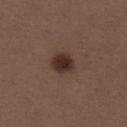Notes:
* follow-up: imaged on a skin check; not biopsied
* subject: female, aged approximately 40
* imaging modality: 15 mm crop, total-body photography
* lesion diameter: ~2.5 mm (longest diameter)
* tile lighting: white-light illumination
* body site: the right thigh